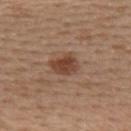patient:
  sex: female
  age_approx: 40
site: upper back
image:
  source: total-body photography crop
  field_of_view_mm: 15
automated_metrics:
  area_mm2_approx: 6.5
  eccentricity: 0.7
  shape_asymmetry: 0.3
  cielab_L: 43
  cielab_a: 21
  cielab_b: 28
  vs_skin_darker_L: 11.0
  vs_skin_contrast_norm: 8.5
  nevus_likeness_0_100: 95